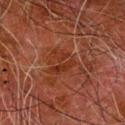Clinical summary: This is a cross-polarized tile. Measured at roughly 3 mm in maximum diameter. From the right forearm. The patient is a male about 80 years old. A close-up tile cropped from a whole-body skin photograph, about 15 mm across. The lesion-visualizer software estimated a nevus-likeness score of about 0/100.